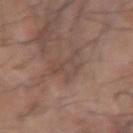<record>
  <site>arm</site>
  <image>
    <source>total-body photography crop</source>
    <field_of_view_mm>15</field_of_view_mm>
  </image>
  <patient>
    <sex>male</sex>
    <age_approx>70</age_approx>
  </patient>
</record>